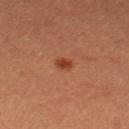This lesion was catalogued during total-body skin photography and was not selected for biopsy. The lesion's longest dimension is about 2 mm. The lesion is on the leg. A roughly 15 mm field-of-view crop from a total-body skin photograph. This is a cross-polarized tile. An algorithmic analysis of the crop reported an outline eccentricity of about 0.7 (0 = round, 1 = elongated) and two-axis asymmetry of about 0.3. And it measured a lesion color around L≈38 a*≈26 b*≈33 in CIELAB, about 9 CIELAB-L* units darker than the surrounding skin, and a lesion-to-skin contrast of about 8 (normalized; higher = more distinct). And it measured a border-irregularity index near 2.5/10, internal color variation of about 2 on a 0–10 scale, and a peripheral color-asymmetry measure near 0.5. A female subject, about 30 years old.